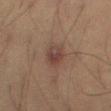The lesion was photographed on a routine skin check and not biopsied; there is no pathology result. An algorithmic analysis of the crop reported a lesion area of about 5.5 mm², an eccentricity of roughly 0.6, and a symmetry-axis asymmetry near 0.2. It also reported a mean CIELAB color near L≈35 a*≈17 b*≈20, roughly 7 lightness units darker than nearby skin, and a normalized border contrast of about 7. The software also gave border irregularity of about 1.5 on a 0–10 scale, a color-variation rating of about 5/10, and radial color variation of about 1.5. A male subject about 55 years old. A roughly 15 mm field-of-view crop from a total-body skin photograph. The tile uses cross-polarized illumination. The recorded lesion diameter is about 3 mm. The lesion is on the left thigh.